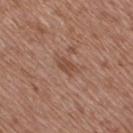workup: no biopsy performed (imaged during a skin exam)
automated lesion analysis: an automated nevus-likeness rating near 0 out of 100
image: 15 mm crop, total-body photography
tile lighting: white-light illumination
size: ~2.5 mm (longest diameter)
subject: female, approximately 75 years of age
location: the right thigh